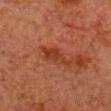The lesion was photographed on a routine skin check and not biopsied; there is no pathology result.
Approximately 4.5 mm at its widest.
On the chest.
A roughly 15 mm field-of-view crop from a total-body skin photograph.
Automated tile analysis of the lesion measured a footprint of about 5.5 mm² and two-axis asymmetry of about 0.4. The analysis additionally found a border-irregularity index near 5.5/10. And it measured a lesion-detection confidence of about 100/100.
The subject is a male aged 78 to 82.